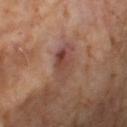follow-up — imaged on a skin check; not biopsied | image source — 15 mm crop, total-body photography | subject — male, in their mid-60s.